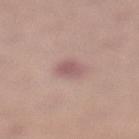Q: Was this lesion biopsied?
A: catalogued during a skin exam; not biopsied
Q: Who is the patient?
A: female, roughly 65 years of age
Q: Lesion location?
A: the left lower leg
Q: What kind of image is this?
A: 15 mm crop, total-body photography